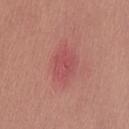Recorded during total-body skin imaging; not selected for excision or biopsy.
A female subject, aged 23 to 27.
The recorded lesion diameter is about 4 mm.
Located on the lower back.
Imaged with white-light lighting.
A 15 mm crop from a total-body photograph taken for skin-cancer surveillance.